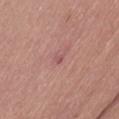A region of skin cropped from a whole-body photographic capture, roughly 15 mm wide. Longest diameter approximately 1 mm. The lesion-visualizer software estimated a lesion area of about 1 mm², an outline eccentricity of about 0.5 (0 = round, 1 = elongated), and a shape-asymmetry score of about 0.5 (0 = symmetric). And it measured a within-lesion color-variation index near 0/10 and radial color variation of about 0. The analysis additionally found a lesion-detection confidence of about 100/100. Located on the left thigh. The subject is a female aged 58 to 62. This is a white-light tile.